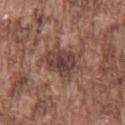<case>
  <biopsy_status>not biopsied; imaged during a skin examination</biopsy_status>
  <lesion_size>
    <long_diameter_mm_approx>4.5</long_diameter_mm_approx>
  </lesion_size>
  <image>
    <source>total-body photography crop</source>
    <field_of_view_mm>15</field_of_view_mm>
  </image>
  <patient>
    <sex>male</sex>
    <age_approx>75</age_approx>
  </patient>
  <site>front of the torso</site>
</case>